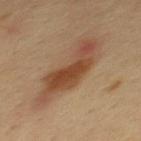biopsy_status: not biopsied; imaged during a skin examination
patient:
  sex: male
  age_approx: 35
lesion_size:
  long_diameter_mm_approx: 8.0
lighting: cross-polarized
site: mid back
image:
  source: total-body photography crop
  field_of_view_mm: 15